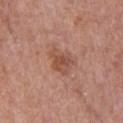Q: Was a biopsy performed?
A: imaged on a skin check; not biopsied
Q: Who is the patient?
A: male, approximately 70 years of age
Q: Automated lesion metrics?
A: an area of roughly 6.5 mm² and an outline eccentricity of about 0.65 (0 = round, 1 = elongated); border irregularity of about 3 on a 0–10 scale, internal color variation of about 4 on a 0–10 scale, and radial color variation of about 1.5
Q: What is the anatomic site?
A: the chest
Q: Illumination type?
A: white-light illumination
Q: How was this image acquired?
A: total-body-photography crop, ~15 mm field of view